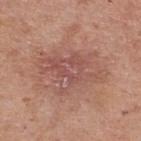The lesion was photographed on a routine skin check and not biopsied; there is no pathology result. The total-body-photography lesion software estimated a lesion color around L≈53 a*≈23 b*≈25 in CIELAB and a normalized border contrast of about 5.5. The analysis additionally found border irregularity of about 7 on a 0–10 scale, internal color variation of about 5 on a 0–10 scale, and radial color variation of about 1.5. And it measured an automated nevus-likeness rating near 0 out of 100 and lesion-presence confidence of about 100/100. A male subject aged 68 to 72. Cropped from a total-body skin-imaging series; the visible field is about 15 mm. About 7.5 mm across. The lesion is on the upper back. Imaged with white-light lighting.